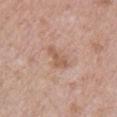Captured during whole-body skin photography for melanoma surveillance; the lesion was not biopsied. Cropped from a whole-body photographic skin survey; the tile spans about 15 mm. The patient is a male aged 48–52. The lesion is located on the chest.